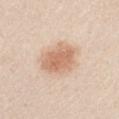The lesion was tiled from a total-body skin photograph and was not biopsied. Automated image analysis of the tile measured a mean CIELAB color near L≈67 a*≈19 b*≈32, roughly 11 lightness units darker than nearby skin, and a normalized lesion–skin contrast near 7.5. The software also gave a within-lesion color-variation index near 3.5/10. This is a white-light tile. From the right upper arm. Measured at roughly 5 mm in maximum diameter. A 15 mm crop from a total-body photograph taken for skin-cancer surveillance. A male patient aged approximately 45.